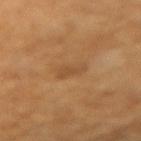| feature | finding |
|---|---|
| biopsy status | catalogued during a skin exam; not biopsied |
| site | the left forearm |
| image-analysis metrics | a lesion area of about 4.5 mm², a shape eccentricity near 0.85, and two-axis asymmetry of about 0.3; a border-irregularity index near 3/10, internal color variation of about 1.5 on a 0–10 scale, and radial color variation of about 0.5; an automated nevus-likeness rating near 0 out of 100 |
| lighting | cross-polarized |
| imaging modality | ~15 mm tile from a whole-body skin photo |
| size | about 3 mm |
| subject | female, aged 58–62 |